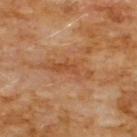| key | value |
|---|---|
| workup | catalogued during a skin exam; not biopsied |
| automated lesion analysis | a footprint of about 9.5 mm², a shape eccentricity near 0.95, and a shape-asymmetry score of about 0.4 (0 = symmetric); a classifier nevus-likeness of about 0/100 |
| size | about 6 mm |
| subject | male, aged approximately 60 |
| imaging modality | ~15 mm tile from a whole-body skin photo |
| body site | the chest |